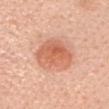Notes:
* notes · total-body-photography surveillance lesion; no biopsy
* body site · the head or neck
* subject · female, in their 40s
* imaging modality · ~15 mm crop, total-body skin-cancer survey
* lesion size · ≈5 mm
* illumination · white-light illumination